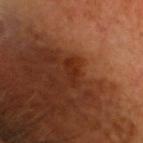{
  "biopsy_status": "not biopsied; imaged during a skin examination",
  "lesion_size": {
    "long_diameter_mm_approx": 3.0
  },
  "patient": {
    "sex": "male",
    "age_approx": 60
  },
  "image": {
    "source": "total-body photography crop",
    "field_of_view_mm": 15
  },
  "site": "head or neck",
  "automated_metrics": {
    "border_irregularity_0_10": 4.0,
    "color_variation_0_10": 0.0,
    "peripheral_color_asymmetry": 0.0,
    "nevus_likeness_0_100": 0
  }
}